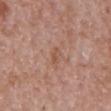<lesion>
<biopsy_status>not biopsied; imaged during a skin examination</biopsy_status>
<patient>
  <sex>male</sex>
  <age_approx>65</age_approx>
</patient>
<image>
  <source>total-body photography crop</source>
  <field_of_view_mm>15</field_of_view_mm>
</image>
<site>chest</site>
<lesion_size>
  <long_diameter_mm_approx>2.5</long_diameter_mm_approx>
</lesion_size>
</lesion>